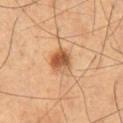Q: Was this lesion biopsied?
A: no biopsy performed (imaged during a skin exam)
Q: Lesion size?
A: ≈4.5 mm
Q: Patient demographics?
A: male, aged approximately 60
Q: Lesion location?
A: the left thigh
Q: How was this image acquired?
A: ~15 mm crop, total-body skin-cancer survey